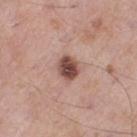Impression:
Recorded during total-body skin imaging; not selected for excision or biopsy.
Context:
A lesion tile, about 15 mm wide, cut from a 3D total-body photograph. Captured under white-light illumination. The lesion's longest dimension is about 3 mm. Located on the left thigh. A male subject in their mid-50s.